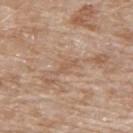Recorded during total-body skin imaging; not selected for excision or biopsy. A male patient aged approximately 80. A region of skin cropped from a whole-body photographic capture, roughly 15 mm wide. Located on the upper back.